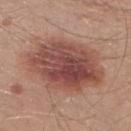Clinical impression: The lesion was tiled from a total-body skin photograph and was not biopsied. Background: The patient is a male aged around 30. The lesion's longest dimension is about 9 mm. On the right lower leg. Automated image analysis of the tile measured a lesion color around L≈48 a*≈23 b*≈25 in CIELAB, about 13 CIELAB-L* units darker than the surrounding skin, and a lesion-to-skin contrast of about 9 (normalized; higher = more distinct). This image is a 15 mm lesion crop taken from a total-body photograph.